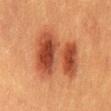biopsy status=imaged on a skin check; not biopsied
diameter=~6.5 mm (longest diameter)
tile lighting=cross-polarized
anatomic site=the mid back
imaging modality=total-body-photography crop, ~15 mm field of view
patient=male, approximately 40 years of age
TBP lesion metrics=a mean CIELAB color near L≈41 a*≈25 b*≈32, roughly 11 lightness units darker than nearby skin, and a normalized border contrast of about 9; a border-irregularity index near 4.5/10, internal color variation of about 9 on a 0–10 scale, and a peripheral color-asymmetry measure near 3; a nevus-likeness score of about 100/100 and a lesion-detection confidence of about 100/100